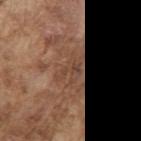The lesion was tiled from a total-body skin photograph and was not biopsied.